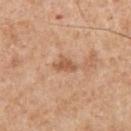| feature | finding |
|---|---|
| notes | catalogued during a skin exam; not biopsied |
| patient | male, in their 60s |
| tile lighting | white-light |
| size | ≈3 mm |
| body site | the right upper arm |
| image | ~15 mm tile from a whole-body skin photo |